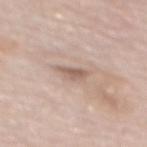Impression:
Part of a total-body skin-imaging series; this lesion was reviewed on a skin check and was not flagged for biopsy.
Acquisition and patient details:
The subject is a female aged around 65. On the mid back. A 15 mm close-up tile from a total-body photography series done for melanoma screening.